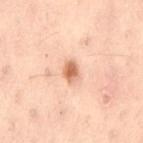The subject is a female aged around 55. Located on the lower back. Cropped from a total-body skin-imaging series; the visible field is about 15 mm.